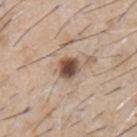Captured during whole-body skin photography for melanoma surveillance; the lesion was not biopsied. On the chest. A male subject, aged 58 to 62. About 3 mm across. The lesion-visualizer software estimated a border-irregularity index near 1.5/10, a within-lesion color-variation index near 5/10, and peripheral color asymmetry of about 1.5. It also reported a detector confidence of about 100 out of 100 that the crop contains a lesion. Captured under white-light illumination. A 15 mm crop from a total-body photograph taken for skin-cancer surveillance.